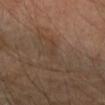| key | value |
|---|---|
| workup | total-body-photography surveillance lesion; no biopsy |
| site | the right forearm |
| automated lesion analysis | a lesion area of about 1 mm², an outline eccentricity of about 0.85 (0 = round, 1 = elongated), and two-axis asymmetry of about 0.45; an average lesion color of about L≈38 a*≈15 b*≈27 (CIELAB) and a lesion-to-skin contrast of about 3.5 (normalized; higher = more distinct); border irregularity of about 3 on a 0–10 scale and a within-lesion color-variation index near 0/10; a nevus-likeness score of about 0/100 and a detector confidence of about 90 out of 100 that the crop contains a lesion |
| image source | total-body-photography crop, ~15 mm field of view |
| tile lighting | cross-polarized illumination |
| diameter | about 1 mm |
| subject | male, aged around 55 |